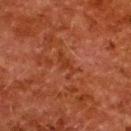Impression:
Recorded during total-body skin imaging; not selected for excision or biopsy.
Image and clinical context:
The lesion is located on the upper back. A 15 mm crop from a total-body photograph taken for skin-cancer surveillance. A female patient, aged approximately 50. About 3.5 mm across.